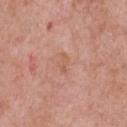This lesion was catalogued during total-body skin photography and was not selected for biopsy.
Measured at roughly 2.5 mm in maximum diameter.
A male subject aged 48–52.
Automated tile analysis of the lesion measured an automated nevus-likeness rating near 0 out of 100 and a detector confidence of about 100 out of 100 that the crop contains a lesion.
A roughly 15 mm field-of-view crop from a total-body skin photograph.
On the chest.